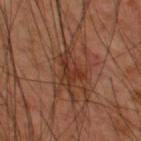{
  "biopsy_status": "not biopsied; imaged during a skin examination",
  "lesion_size": {
    "long_diameter_mm_approx": 4.5
  },
  "image": {
    "source": "total-body photography crop",
    "field_of_view_mm": 15
  },
  "patient": {
    "sex": "male",
    "age_approx": 60
  },
  "site": "upper back",
  "automated_metrics": {
    "area_mm2_approx": 8.0,
    "eccentricity": 0.75,
    "shape_asymmetry": 0.55,
    "border_irregularity_0_10": 6.0,
    "peripheral_color_asymmetry": 1.5
  },
  "lighting": "cross-polarized"
}